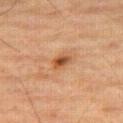Captured during whole-body skin photography for melanoma surveillance; the lesion was not biopsied.
A male subject, in their mid- to late 80s or older.
Located on the right thigh.
This is a cross-polarized tile.
About 2.5 mm across.
A 15 mm close-up extracted from a 3D total-body photography capture.
The lesion-visualizer software estimated a border-irregularity index near 2.5/10.